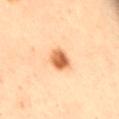Impression: Recorded during total-body skin imaging; not selected for excision or biopsy. Context: The lesion is located on the leg. A 15 mm close-up extracted from a 3D total-body photography capture. A male subject in their 50s.